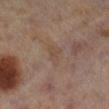The lesion is located on the right lower leg.
About 3.5 mm across.
Automated image analysis of the tile measured a lesion area of about 4.5 mm² and a shape eccentricity near 0.85.
A female patient, approximately 40 years of age.
This is a cross-polarized tile.
A lesion tile, about 15 mm wide, cut from a 3D total-body photograph.